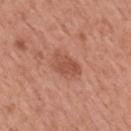Imaged during a routine full-body skin examination; the lesion was not biopsied and no histopathology is available.
A lesion tile, about 15 mm wide, cut from a 3D total-body photograph.
Longest diameter approximately 4 mm.
Captured under white-light illumination.
A male subject in their mid-50s.
From the mid back.
Automated image analysis of the tile measured a mean CIELAB color near L≈52 a*≈26 b*≈31 and a normalized border contrast of about 6.5.